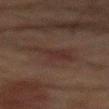  biopsy_status: not biopsied; imaged during a skin examination
  image:
    source: total-body photography crop
    field_of_view_mm: 15
  lesion_size:
    long_diameter_mm_approx: 4.5
  lighting: cross-polarized
  patient:
    sex: male
    age_approx: 65
  site: back